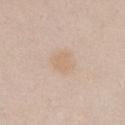Notes:
* workup · no biopsy performed (imaged during a skin exam)
* imaging modality · ~15 mm crop, total-body skin-cancer survey
* subject · female, aged around 25
* site · the chest